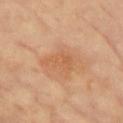Assessment:
The lesion was photographed on a routine skin check and not biopsied; there is no pathology result.
Acquisition and patient details:
Located on the left upper arm. The patient is a female approximately 75 years of age. A roughly 15 mm field-of-view crop from a total-body skin photograph.